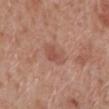Q: Was a biopsy performed?
A: imaged on a skin check; not biopsied
Q: Who is the patient?
A: male, roughly 70 years of age
Q: How was this image acquired?
A: ~15 mm crop, total-body skin-cancer survey
Q: Automated lesion metrics?
A: a footprint of about 6.5 mm², an eccentricity of roughly 0.65, and two-axis asymmetry of about 0.25; a normalized lesion–skin contrast near 5.5; a classifier nevus-likeness of about 15/100 and lesion-presence confidence of about 100/100
Q: Illumination type?
A: white-light illumination
Q: Where on the body is the lesion?
A: the leg